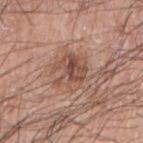The lesion was photographed on a routine skin check and not biopsied; there is no pathology result.
From the left arm.
The patient is a male about 70 years old.
The tile uses white-light illumination.
A 15 mm close-up tile from a total-body photography series done for melanoma screening.